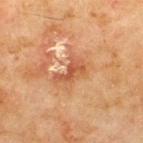The lesion was photographed on a routine skin check and not biopsied; there is no pathology result. Imaged with cross-polarized lighting. The lesion is on the upper back. A male subject, aged approximately 70. Measured at roughly 3.5 mm in maximum diameter. A roughly 15 mm field-of-view crop from a total-body skin photograph.